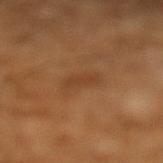{
  "biopsy_status": "not biopsied; imaged during a skin examination",
  "patient": {
    "sex": "male",
    "age_approx": 65
  },
  "image": {
    "source": "total-body photography crop",
    "field_of_view_mm": 15
  },
  "site": "left lower leg"
}